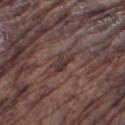Case summary:
* subject — male, about 75 years old
* image — total-body-photography crop, ~15 mm field of view
* lesion diameter — about 3 mm
* illumination — white-light
* anatomic site — the leg
* automated metrics — a footprint of about 3.5 mm², a shape eccentricity near 0.85, and a shape-asymmetry score of about 0.45 (0 = symmetric); an average lesion color of about L≈33 a*≈17 b*≈17 (CIELAB) and a lesion–skin lightness drop of about 8